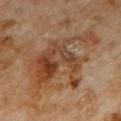| key | value |
|---|---|
| biopsy status | imaged on a skin check; not biopsied |
| subject | male, roughly 60 years of age |
| diameter | ~10.5 mm (longest diameter) |
| lighting | cross-polarized |
| image-analysis metrics | a lesion color around L≈42 a*≈20 b*≈32 in CIELAB and a normalized border contrast of about 8; a classifier nevus-likeness of about 0/100 and lesion-presence confidence of about 100/100 |
| body site | the chest |
| acquisition | 15 mm crop, total-body photography |